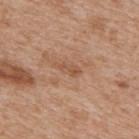follow-up: imaged on a skin check; not biopsied | body site: the upper back | lighting: white-light | acquisition: 15 mm crop, total-body photography | subject: male, approximately 65 years of age.